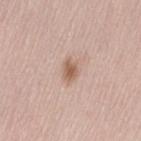Part of a total-body skin-imaging series; this lesion was reviewed on a skin check and was not flagged for biopsy.
The lesion is located on the left thigh.
The subject is a female in their mid- to late 60s.
The tile uses white-light illumination.
A 15 mm close-up tile from a total-body photography series done for melanoma screening.
About 2.5 mm across.